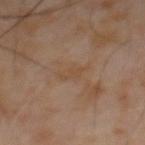imaging modality=~15 mm tile from a whole-body skin photo
patient=male, in their mid-60s